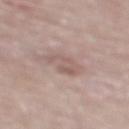Part of a total-body skin-imaging series; this lesion was reviewed on a skin check and was not flagged for biopsy.
A region of skin cropped from a whole-body photographic capture, roughly 15 mm wide.
Located on the mid back.
The subject is a male aged 78 to 82.
The tile uses white-light illumination.
Measured at roughly 3 mm in maximum diameter.